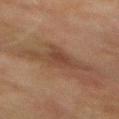<case>
<biopsy_status>not biopsied; imaged during a skin examination</biopsy_status>
<lighting>cross-polarized</lighting>
<lesion_size>
  <long_diameter_mm_approx>5.5</long_diameter_mm_approx>
</lesion_size>
<site>mid back</site>
<image>
  <source>total-body photography crop</source>
  <field_of_view_mm>15</field_of_view_mm>
</image>
<patient>
  <sex>male</sex>
  <age_approx>75</age_approx>
</patient>
</case>